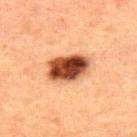Assessment: The lesion was tiled from a total-body skin photograph and was not biopsied. Image and clinical context: A 15 mm close-up tile from a total-body photography series done for melanoma screening. A male subject, in their 50s. Automated image analysis of the tile measured a mean CIELAB color near L≈38 a*≈23 b*≈31, a lesion–skin lightness drop of about 21, and a lesion-to-skin contrast of about 15.5 (normalized; higher = more distinct). It also reported an automated nevus-likeness rating near 100 out of 100 and a lesion-detection confidence of about 100/100. Captured under cross-polarized illumination. Located on the upper back. The recorded lesion diameter is about 5 mm.